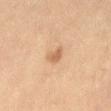Part of a total-body skin-imaging series; this lesion was reviewed on a skin check and was not flagged for biopsy. Imaged with cross-polarized lighting. The lesion's longest dimension is about 3 mm. A female subject in their 60s. The lesion is on the abdomen. This image is a 15 mm lesion crop taken from a total-body photograph.